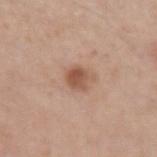notes: total-body-photography surveillance lesion; no biopsy
image: 15 mm crop, total-body photography
image-analysis metrics: a mean CIELAB color near L≈54 a*≈21 b*≈30 and about 11 CIELAB-L* units darker than the surrounding skin; a detector confidence of about 100 out of 100 that the crop contains a lesion
diameter: about 3 mm
tile lighting: white-light
site: the left upper arm
patient: male, in their 60s